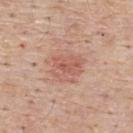The recorded lesion diameter is about 3.5 mm. A region of skin cropped from a whole-body photographic capture, roughly 15 mm wide. The total-body-photography lesion software estimated a border-irregularity rating of about 3.5/10 and radial color variation of about 1.5. And it measured an automated nevus-likeness rating near 25 out of 100 and lesion-presence confidence of about 100/100. A male patient about 55 years old. The lesion is on the upper back.